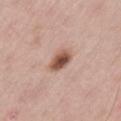<lesion>
<biopsy_status>not biopsied; imaged during a skin examination</biopsy_status>
<site>leg</site>
<image>
  <source>total-body photography crop</source>
  <field_of_view_mm>15</field_of_view_mm>
</image>
<lesion_size>
  <long_diameter_mm_approx>3.0</long_diameter_mm_approx>
</lesion_size>
<patient>
  <sex>male</sex>
  <age_approx>60</age_approx>
</patient>
<lighting>white-light</lighting>
</lesion>